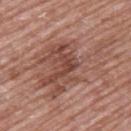Q: Is there a histopathology result?
A: imaged on a skin check; not biopsied
Q: How was the tile lit?
A: white-light
Q: Who is the patient?
A: female, roughly 60 years of age
Q: What is the lesion's diameter?
A: about 11 mm
Q: Lesion location?
A: the upper back
Q: What kind of image is this?
A: ~15 mm crop, total-body skin-cancer survey